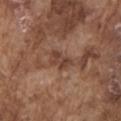Q: Was a biopsy performed?
A: imaged on a skin check; not biopsied
Q: Automated lesion metrics?
A: an area of roughly 5 mm² and an outline eccentricity of about 0.8 (0 = round, 1 = elongated); border irregularity of about 3 on a 0–10 scale, a color-variation rating of about 3/10, and a peripheral color-asymmetry measure near 1
Q: What is the imaging modality?
A: ~15 mm crop, total-body skin-cancer survey
Q: What is the lesion's diameter?
A: ≈3 mm
Q: What is the anatomic site?
A: the left upper arm
Q: Patient demographics?
A: male, in their mid-70s